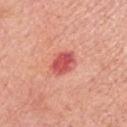The lesion's longest dimension is about 3.5 mm. On the right upper arm. The patient is a female aged 63–67. Captured under white-light illumination. A region of skin cropped from a whole-body photographic capture, roughly 15 mm wide.